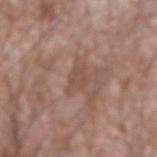Impression: No biopsy was performed on this lesion — it was imaged during a full skin examination and was not determined to be concerning. Acquisition and patient details: Captured under white-light illumination. The subject is a male roughly 60 years of age. A roughly 15 mm field-of-view crop from a total-body skin photograph. An algorithmic analysis of the crop reported a lesion color around L≈49 a*≈18 b*≈25 in CIELAB and a normalized lesion–skin contrast near 5. The software also gave lesion-presence confidence of about 65/100. On the left forearm. The recorded lesion diameter is about 3.5 mm.